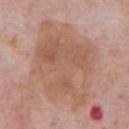workup: no biopsy performed (imaged during a skin exam)
patient: male, aged approximately 60
TBP lesion metrics: a lesion color around L≈57 a*≈20 b*≈29 in CIELAB, roughly 8 lightness units darker than nearby skin, and a lesion-to-skin contrast of about 6 (normalized; higher = more distinct); a classifier nevus-likeness of about 0/100
image: ~15 mm crop, total-body skin-cancer survey
site: the front of the torso
lighting: white-light
lesion size: ≈9.5 mm